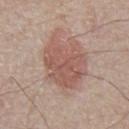notes: no biopsy performed (imaged during a skin exam) | image source: ~15 mm crop, total-body skin-cancer survey | lighting: white-light | body site: the abdomen | lesion diameter: about 6 mm | patient: male, aged around 65 | image-analysis metrics: an area of roughly 23 mm², a shape eccentricity near 0.6, and a shape-asymmetry score of about 0.2 (0 = symmetric); about 9 CIELAB-L* units darker than the surrounding skin; a border-irregularity rating of about 3/10, a within-lesion color-variation index near 3.5/10, and radial color variation of about 1; an automated nevus-likeness rating near 90 out of 100 and a lesion-detection confidence of about 100/100.